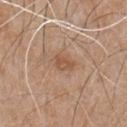{
  "biopsy_status": "not biopsied; imaged during a skin examination",
  "lesion_size": {
    "long_diameter_mm_approx": 2.5
  },
  "patient": {
    "sex": "male",
    "age_approx": 65
  },
  "lighting": "white-light",
  "site": "chest",
  "image": {
    "source": "total-body photography crop",
    "field_of_view_mm": 15
  }
}